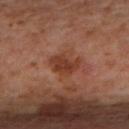No biopsy was performed on this lesion — it was imaged during a full skin examination and was not determined to be concerning.
The lesion-visualizer software estimated a nevus-likeness score of about 0/100.
A female subject, aged around 45.
Located on the back.
A close-up tile cropped from a whole-body skin photograph, about 15 mm across.